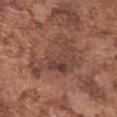Clinical impression:
The lesion was photographed on a routine skin check and not biopsied; there is no pathology result.
Context:
Imaged with white-light lighting. A male subject aged 73 to 77. Located on the left upper arm. Approximately 7 mm at its widest. A 15 mm close-up tile from a total-body photography series done for melanoma screening.